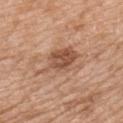<record>
  <biopsy_status>not biopsied; imaged during a skin examination</biopsy_status>
  <image>
    <source>total-body photography crop</source>
    <field_of_view_mm>15</field_of_view_mm>
  </image>
  <lighting>white-light</lighting>
  <patient>
    <sex>male</sex>
    <age_approx>50</age_approx>
  </patient>
  <site>upper back</site>
  <automated_metrics>
    <nevus_likeness_0_100>55</nevus_likeness_0_100>
    <lesion_detection_confidence_0_100>100</lesion_detection_confidence_0_100>
  </automated_metrics>
</record>